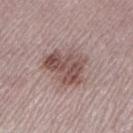Imaged during a routine full-body skin examination; the lesion was not biopsied and no histopathology is available.
Longest diameter approximately 5.5 mm.
Cropped from a total-body skin-imaging series; the visible field is about 15 mm.
A female subject approximately 50 years of age.
An algorithmic analysis of the crop reported a nevus-likeness score of about 55/100 and a lesion-detection confidence of about 100/100.
The tile uses white-light illumination.
From the left lower leg.